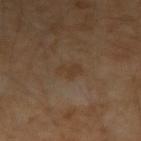follow-up: total-body-photography surveillance lesion; no biopsy
subject: male, aged approximately 45
image-analysis metrics: a lesion area of about 4 mm²
illumination: cross-polarized illumination
diameter: ~2.5 mm (longest diameter)
location: the arm
image source: 15 mm crop, total-body photography